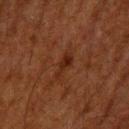image = 15 mm crop, total-body photography | patient = male, about 60 years old | size = ~3.5 mm (longest diameter) | automated metrics = a footprint of about 4 mm², a shape eccentricity near 0.9, and a symmetry-axis asymmetry near 0.4; a mean CIELAB color near L≈20 a*≈19 b*≈23, roughly 5 lightness units darker than nearby skin, and a normalized lesion–skin contrast near 7; border irregularity of about 4.5 on a 0–10 scale and radial color variation of about 0 | body site = the upper back.